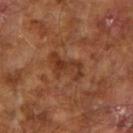Assessment: Part of a total-body skin-imaging series; this lesion was reviewed on a skin check and was not flagged for biopsy. Context: On the right upper arm. A male patient aged 63 to 67. An algorithmic analysis of the crop reported an outline eccentricity of about 0.85 (0 = round, 1 = elongated) and a symmetry-axis asymmetry near 0.55. It also reported a border-irregularity rating of about 7/10 and peripheral color asymmetry of about 0.5. A 15 mm close-up tile from a total-body photography series done for melanoma screening. Measured at roughly 4.5 mm in maximum diameter.